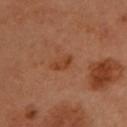A lesion tile, about 15 mm wide, cut from a 3D total-body photograph.
Located on the head or neck.
Imaged with cross-polarized lighting.
An algorithmic analysis of the crop reported a border-irregularity index near 3.5/10 and a within-lesion color-variation index near 0/10. The analysis additionally found a lesion-detection confidence of about 100/100.
The subject is a female aged 58 to 62.